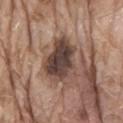– follow-up — no biopsy performed (imaged during a skin exam)
– illumination — white-light illumination
– subject — male, aged approximately 80
– imaging modality — ~15 mm tile from a whole-body skin photo
– automated lesion analysis — an automated nevus-likeness rating near 10 out of 100
– anatomic site — the lower back